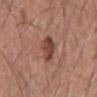notes — catalogued during a skin exam; not biopsied
body site — the mid back
subject — male, aged 53–57
diameter — about 3.5 mm
imaging modality — ~15 mm crop, total-body skin-cancer survey